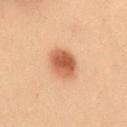Q: Is there a histopathology result?
A: no biopsy performed (imaged during a skin exam)
Q: Where on the body is the lesion?
A: the front of the torso
Q: What is the imaging modality?
A: 15 mm crop, total-body photography
Q: How large is the lesion?
A: ~4 mm (longest diameter)
Q: Illumination type?
A: cross-polarized illumination
Q: Automated lesion metrics?
A: an average lesion color of about L≈47 a*≈22 b*≈30 (CIELAB), roughly 13 lightness units darker than nearby skin, and a normalized lesion–skin contrast near 9; a classifier nevus-likeness of about 100/100 and lesion-presence confidence of about 100/100
Q: Who is the patient?
A: female, about 40 years old